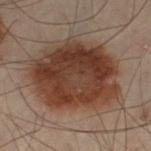The lesion's longest dimension is about 9 mm. The patient is a male in their 50s. This image is a 15 mm lesion crop taken from a total-body photograph. Captured under cross-polarized illumination. On the left thigh.